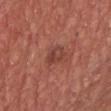Impression: Part of a total-body skin-imaging series; this lesion was reviewed on a skin check and was not flagged for biopsy. Background: The lesion-visualizer software estimated an eccentricity of roughly 0.75 and two-axis asymmetry of about 0.3. And it measured a lesion color around L≈42 a*≈26 b*≈27 in CIELAB. The software also gave a within-lesion color-variation index near 5/10 and peripheral color asymmetry of about 2. A male patient, aged approximately 65. A 15 mm crop from a total-body photograph taken for skin-cancer surveillance. Located on the chest. About 3 mm across.